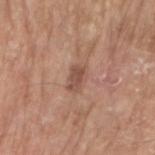  biopsy_status: not biopsied; imaged during a skin examination
  patient:
    sex: male
    age_approx: 80
  site: right upper arm
  image:
    source: total-body photography crop
    field_of_view_mm: 15
  lesion_size:
    long_diameter_mm_approx: 3.0
  lighting: white-light